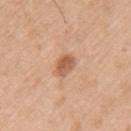{
  "biopsy_status": "not biopsied; imaged during a skin examination",
  "site": "left upper arm",
  "image": {
    "source": "total-body photography crop",
    "field_of_view_mm": 15
  },
  "automated_metrics": {
    "vs_skin_darker_L": 12.0,
    "border_irregularity_0_10": 1.5,
    "peripheral_color_asymmetry": 1.5,
    "nevus_likeness_0_100": 75,
    "lesion_detection_confidence_0_100": 100
  },
  "lesion_size": {
    "long_diameter_mm_approx": 2.5
  },
  "lighting": "white-light",
  "patient": {
    "sex": "male",
    "age_approx": 70
  }
}